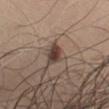Clinical impression:
This lesion was catalogued during total-body skin photography and was not selected for biopsy.
Image and clinical context:
The subject is a male in their mid- to late 50s. This image is a 15 mm lesion crop taken from a total-body photograph. Automated tile analysis of the lesion measured border irregularity of about 3 on a 0–10 scale, internal color variation of about 4 on a 0–10 scale, and peripheral color asymmetry of about 1.5. About 2.5 mm across. The lesion is on the left upper arm. This is a white-light tile.